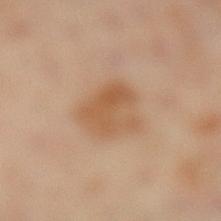Impression: Recorded during total-body skin imaging; not selected for excision or biopsy. Context: This is a cross-polarized tile. Automated tile analysis of the lesion measured a lesion color around L≈57 a*≈20 b*≈36 in CIELAB and a lesion-to-skin contrast of about 7 (normalized; higher = more distinct). A 15 mm crop from a total-body photograph taken for skin-cancer surveillance. The subject is a female aged 43 to 47. Measured at roughly 4 mm in maximum diameter. The lesion is on the left lower leg.